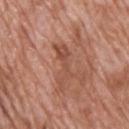Recorded during total-body skin imaging; not selected for excision or biopsy. A male subject aged approximately 70. On the upper back. Cropped from a total-body skin-imaging series; the visible field is about 15 mm. The recorded lesion diameter is about 5 mm. The tile uses white-light illumination.